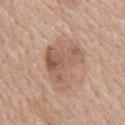<lesion>
<biopsy_status>not biopsied; imaged during a skin examination</biopsy_status>
<image>
  <source>total-body photography crop</source>
  <field_of_view_mm>15</field_of_view_mm>
</image>
<lesion_size>
  <long_diameter_mm_approx>6.5</long_diameter_mm_approx>
</lesion_size>
<automated_metrics>
  <area_mm2_approx>18.0</area_mm2_approx>
  <shape_asymmetry>0.4</shape_asymmetry>
  <border_irregularity_0_10>5.5</border_irregularity_0_10>
  <color_variation_0_10>6.0</color_variation_0_10>
  <peripheral_color_asymmetry>2.5</peripheral_color_asymmetry>
  <nevus_likeness_0_100>20</nevus_likeness_0_100>
  <lesion_detection_confidence_0_100>100</lesion_detection_confidence_0_100>
</automated_metrics>
<patient>
  <sex>male</sex>
  <age_approx>75</age_approx>
</patient>
<site>chest</site>
</lesion>